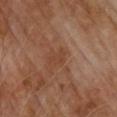Part of a total-body skin-imaging series; this lesion was reviewed on a skin check and was not flagged for biopsy. A male subject, roughly 70 years of age. The lesion is on the upper back. Automated tile analysis of the lesion measured an area of roughly 2.5 mm², an eccentricity of roughly 0.85, and two-axis asymmetry of about 0.45. And it measured a lesion color around L≈41 a*≈21 b*≈30 in CIELAB, roughly 5 lightness units darker than nearby skin, and a normalized border contrast of about 5. And it measured border irregularity of about 5 on a 0–10 scale and radial color variation of about 0. And it measured a nevus-likeness score of about 0/100 and lesion-presence confidence of about 100/100. About 3 mm across. Cropped from a whole-body photographic skin survey; the tile spans about 15 mm. Captured under cross-polarized illumination.